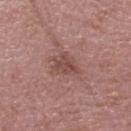Impression: No biopsy was performed on this lesion — it was imaged during a full skin examination and was not determined to be concerning. Image and clinical context: This image is a 15 mm lesion crop taken from a total-body photograph. The lesion's longest dimension is about 3 mm. The patient is a male aged 58–62. The lesion is on the head or neck. An algorithmic analysis of the crop reported a lesion color around L≈47 a*≈22 b*≈23 in CIELAB and a lesion-to-skin contrast of about 6.5 (normalized; higher = more distinct). This is a white-light tile.